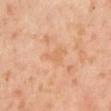subject=male, approximately 30 years of age | acquisition=15 mm crop, total-body photography | TBP lesion metrics=a border-irregularity rating of about 6/10, internal color variation of about 0.5 on a 0–10 scale, and radial color variation of about 0; a detector confidence of about 100 out of 100 that the crop contains a lesion | site=the left arm | lesion size=about 2.5 mm.